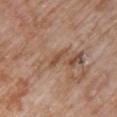Captured during whole-body skin photography for melanoma surveillance; the lesion was not biopsied.
On the chest.
An algorithmic analysis of the crop reported an average lesion color of about L≈50 a*≈20 b*≈30 (CIELAB), a lesion–skin lightness drop of about 8, and a normalized lesion–skin contrast near 6.5.
A female patient, aged 83 or over.
This is a white-light tile.
Cropped from a whole-body photographic skin survey; the tile spans about 15 mm.
About 3 mm across.